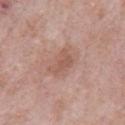This lesion was catalogued during total-body skin photography and was not selected for biopsy. Cropped from a whole-body photographic skin survey; the tile spans about 15 mm. Imaged with white-light lighting. Longest diameter approximately 3.5 mm. The lesion is located on the chest. The subject is a male in their mid- to late 50s. Automated tile analysis of the lesion measured an area of roughly 5.5 mm², a shape eccentricity near 0.8, and a symmetry-axis asymmetry near 0.45. The analysis additionally found a lesion–skin lightness drop of about 7 and a normalized border contrast of about 5.5. The analysis additionally found a border-irregularity rating of about 4.5/10 and radial color variation of about 1. The analysis additionally found a classifier nevus-likeness of about 0/100 and a detector confidence of about 100 out of 100 that the crop contains a lesion.